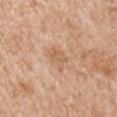Recorded during total-body skin imaging; not selected for excision or biopsy.
A lesion tile, about 15 mm wide, cut from a 3D total-body photograph.
From the left upper arm.
The patient is a male in their mid- to late 60s.
Approximately 3 mm at its widest.